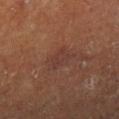follow-up=no biopsy performed (imaged during a skin exam) | patient=female, aged around 80 | location=the left lower leg | image source=~15 mm crop, total-body skin-cancer survey.